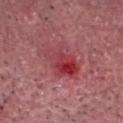The lesion was photographed on a routine skin check and not biopsied; there is no pathology result.
On the chest.
The tile uses white-light illumination.
A male subject aged 83 or older.
A region of skin cropped from a whole-body photographic capture, roughly 15 mm wide.